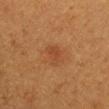Findings:
– follow-up · imaged on a skin check; not biopsied
– illumination · cross-polarized
– body site · the arm
– automated lesion analysis · a mean CIELAB color near L≈36 a*≈21 b*≈30, a lesion–skin lightness drop of about 5, and a lesion-to-skin contrast of about 5 (normalized; higher = more distinct); a border-irregularity index near 3/10, a color-variation rating of about 1.5/10, and peripheral color asymmetry of about 0.5
– patient · female, aged around 40
– image source · 15 mm crop, total-body photography